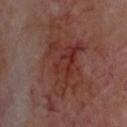• notes — no biopsy performed (imaged during a skin exam)
• imaging modality — 15 mm crop, total-body photography
• body site — the head or neck
• patient — aged approximately 65
• lesion diameter — about 10 mm